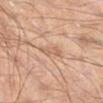A 15 mm close-up tile from a total-body photography series done for melanoma screening. The lesion's longest dimension is about 3.5 mm. Imaged with cross-polarized lighting. Automated tile analysis of the lesion measured a lesion area of about 3.5 mm², an outline eccentricity of about 0.9 (0 = round, 1 = elongated), and two-axis asymmetry of about 0.4. The analysis additionally found a normalized border contrast of about 5. The software also gave a border-irregularity index near 4/10, a within-lesion color-variation index near 1/10, and radial color variation of about 0.5. A male subject about 55 years old. The lesion is located on the right lower leg.